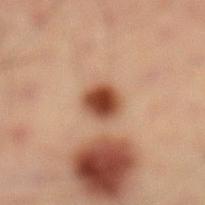The lesion was tiled from a total-body skin photograph and was not biopsied. The subject is a male aged approximately 40. The lesion is located on the left lower leg. A 15 mm crop from a total-body photograph taken for skin-cancer surveillance.